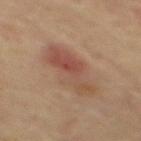The lesion was photographed on a routine skin check and not biopsied; there is no pathology result. A 15 mm crop from a total-body photograph taken for skin-cancer surveillance. The subject is a male about 65 years old. The recorded lesion diameter is about 7 mm. This is a cross-polarized tile. Automated image analysis of the tile measured an average lesion color of about L≈44 a*≈18 b*≈25 (CIELAB), about 8 CIELAB-L* units darker than the surrounding skin, and a lesion-to-skin contrast of about 6.5 (normalized; higher = more distinct). The analysis additionally found a border-irregularity index near 3.5/10, internal color variation of about 7.5 on a 0–10 scale, and a peripheral color-asymmetry measure near 3. The lesion is located on the mid back.